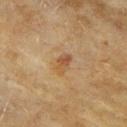Recorded during total-body skin imaging; not selected for excision or biopsy. The subject is a female in their 60s. Imaged with cross-polarized lighting. The lesion is located on the right upper arm. Automated tile analysis of the lesion measured a shape eccentricity near 0.75. It also reported a mean CIELAB color near L≈49 a*≈18 b*≈34 and a normalized lesion–skin contrast near 6. The software also gave internal color variation of about 6 on a 0–10 scale and radial color variation of about 1.5. The software also gave a lesion-detection confidence of about 100/100. Measured at roughly 3 mm in maximum diameter. Cropped from a whole-body photographic skin survey; the tile spans about 15 mm.